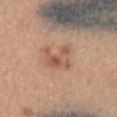* lesion diameter — about 3.5 mm
* imaging modality — ~15 mm tile from a whole-body skin photo
* body site — the mid back
* tile lighting — white-light
* patient — female, about 40 years old